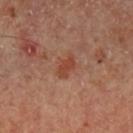Q: Is there a histopathology result?
A: catalogued during a skin exam; not biopsied
Q: Lesion size?
A: about 3 mm
Q: What lighting was used for the tile?
A: cross-polarized
Q: Lesion location?
A: the left lower leg
Q: What did automated image analysis measure?
A: an area of roughly 4 mm²; about 8 CIELAB-L* units darker than the surrounding skin and a lesion-to-skin contrast of about 7 (normalized; higher = more distinct)
Q: What kind of image is this?
A: ~15 mm tile from a whole-body skin photo
Q: Patient demographics?
A: male, about 65 years old